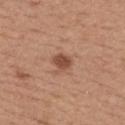The lesion was tiled from a total-body skin photograph and was not biopsied.
On the upper back.
A female subject in their mid- to late 30s.
Imaged with white-light lighting.
A roughly 15 mm field-of-view crop from a total-body skin photograph.